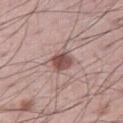Case summary:
* notes · no biopsy performed (imaged during a skin exam)
* lighting · white-light
* site · the right thigh
* patient · male, roughly 60 years of age
* image source · ~15 mm tile from a whole-body skin photo
* TBP lesion metrics · a footprint of about 5 mm², a shape eccentricity near 0.45, and a symmetry-axis asymmetry near 0.2; a lesion color around L≈50 a*≈20 b*≈22 in CIELAB; a detector confidence of about 100 out of 100 that the crop contains a lesion
* lesion size · ~2.5 mm (longest diameter)